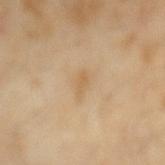illumination: cross-polarized illumination
imaging modality: ~15 mm tile from a whole-body skin photo
patient: male, in their mid-50s
site: the mid back